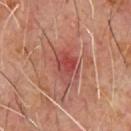<tbp_lesion>
<biopsy_status>not biopsied; imaged during a skin examination</biopsy_status>
<patient>
  <sex>male</sex>
  <age_approx>60</age_approx>
</patient>
<automated_metrics>
  <cielab_L>45</cielab_L>
  <cielab_a>28</cielab_a>
  <cielab_b>25</cielab_b>
  <vs_skin_darker_L>8.0</vs_skin_darker_L>
  <vs_skin_contrast_norm>6.5</vs_skin_contrast_norm>
  <nevus_likeness_0_100>5</nevus_likeness_0_100>
  <lesion_detection_confidence_0_100>100</lesion_detection_confidence_0_100>
</automated_metrics>
<lesion_size>
  <long_diameter_mm_approx>4.5</long_diameter_mm_approx>
</lesion_size>
<image>
  <source>total-body photography crop</source>
  <field_of_view_mm>15</field_of_view_mm>
</image>
<site>chest</site>
</tbp_lesion>